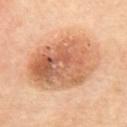  biopsy_status: not biopsied; imaged during a skin examination
  image:
    source: total-body photography crop
    field_of_view_mm: 15
  lighting: cross-polarized
  site: mid back
  lesion_size:
    long_diameter_mm_approx: 10.0
  automated_metrics:
    eccentricity: 0.85
    color_variation_0_10: 9.5
    nevus_likeness_0_100: 50
    lesion_detection_confidence_0_100: 100
  patient:
    sex: female
    age_approx: 60